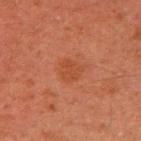follow-up: no biopsy performed (imaged during a skin exam)
automated lesion analysis: a footprint of about 5.5 mm², an outline eccentricity of about 0.3 (0 = round, 1 = elongated), and a symmetry-axis asymmetry near 0.25; a mean CIELAB color near L≈39 a*≈26 b*≈32, roughly 5 lightness units darker than nearby skin, and a normalized border contrast of about 5; a border-irregularity index near 2/10 and internal color variation of about 1.5 on a 0–10 scale; an automated nevus-likeness rating near 0 out of 100 and lesion-presence confidence of about 100/100
subject: male, approximately 45 years of age
location: the right upper arm
image: 15 mm crop, total-body photography
lighting: cross-polarized illumination